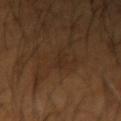Findings:
• workup: catalogued during a skin exam; not biopsied
• patient: male, aged approximately 65
• imaging modality: 15 mm crop, total-body photography
• TBP lesion metrics: a footprint of about 1.5 mm², a shape eccentricity near 0.95, and two-axis asymmetry of about 0.4; a mean CIELAB color near L≈26 a*≈16 b*≈25 and a normalized border contrast of about 4.5; border irregularity of about 5 on a 0–10 scale and a color-variation rating of about 0/10
• body site: the left forearm
• lighting: cross-polarized illumination
• diameter: about 2.5 mm